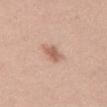| key | value |
|---|---|
| follow-up | no biopsy performed (imaged during a skin exam) |
| image source | ~15 mm tile from a whole-body skin photo |
| lesion size | about 3.5 mm |
| site | the back |
| automated lesion analysis | roughly 11 lightness units darker than nearby skin and a lesion-to-skin contrast of about 7 (normalized; higher = more distinct); a border-irregularity index near 3.5/10, a color-variation rating of about 3/10, and peripheral color asymmetry of about 1; an automated nevus-likeness rating near 30 out of 100 |
| patient | female, aged 33–37 |